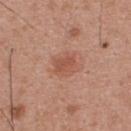{"biopsy_status": "not biopsied; imaged during a skin examination", "patient": {"sex": "male", "age_approx": 30}, "site": "upper back", "image": {"source": "total-body photography crop", "field_of_view_mm": 15}}